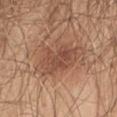This lesion was catalogued during total-body skin photography and was not selected for biopsy. The patient is a male aged approximately 50. A 15 mm close-up tile from a total-body photography series done for melanoma screening. The lesion is on the abdomen.